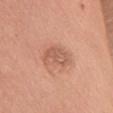workup: no biopsy performed (imaged during a skin exam) | site: the arm | automated lesion analysis: an average lesion color of about L≈58 a*≈24 b*≈31 (CIELAB), roughly 9 lightness units darker than nearby skin, and a normalized lesion–skin contrast near 5.5 | patient: female, approximately 75 years of age | illumination: white-light illumination | imaging modality: ~15 mm tile from a whole-body skin photo | diameter: ~4 mm (longest diameter).